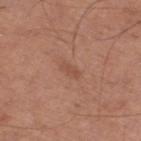{
  "site": "right thigh",
  "patient": {
    "sex": "male",
    "age_approx": 65
  },
  "image": {
    "source": "total-body photography crop",
    "field_of_view_mm": 15
  }
}